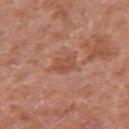Findings:
• biopsy status — imaged on a skin check; not biopsied
• patient — male, roughly 65 years of age
• imaging modality — 15 mm crop, total-body photography
• lesion size — about 3 mm
• lighting — white-light illumination
• location — the arm